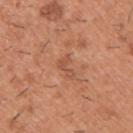workup — imaged on a skin check; not biopsied
subject — male, in their 40s
imaging modality — ~15 mm crop, total-body skin-cancer survey
site — the upper back